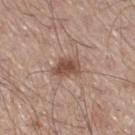Case summary:
- follow-up · catalogued during a skin exam; not biopsied
- lesion size · ≈3 mm
- subject · male, roughly 60 years of age
- body site · the leg
- tile lighting · white-light
- imaging modality · ~15 mm crop, total-body skin-cancer survey